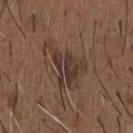Assessment:
Imaged during a routine full-body skin examination; the lesion was not biopsied and no histopathology is available.
Context:
Cropped from a total-body skin-imaging series; the visible field is about 15 mm. The lesion is located on the chest. A male subject in their 50s. Automated image analysis of the tile measured a shape eccentricity near 0.8 and a shape-asymmetry score of about 0.55 (0 = symmetric). It also reported an average lesion color of about L≈36 a*≈16 b*≈23 (CIELAB), a lesion–skin lightness drop of about 8, and a normalized border contrast of about 7.5. The software also gave a border-irregularity rating of about 8/10, internal color variation of about 4 on a 0–10 scale, and a peripheral color-asymmetry measure near 1.5.